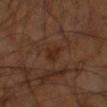{
  "biopsy_status": "not biopsied; imaged during a skin examination",
  "automated_metrics": {
    "area_mm2_approx": 4.0,
    "eccentricity": 0.75,
    "cielab_L": 26,
    "cielab_a": 18,
    "cielab_b": 26,
    "vs_skin_darker_L": 5.0,
    "vs_skin_contrast_norm": 6.5,
    "border_irregularity_0_10": 3.5,
    "color_variation_0_10": 1.5
  },
  "image": {
    "source": "total-body photography crop",
    "field_of_view_mm": 15
  },
  "patient": {
    "sex": "male",
    "age_approx": 60
  },
  "site": "left arm",
  "lighting": "cross-polarized"
}